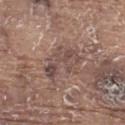Clinical impression: Recorded during total-body skin imaging; not selected for excision or biopsy. Clinical summary: The tile uses white-light illumination. About 5 mm across. The total-body-photography lesion software estimated a footprint of about 11 mm², a shape eccentricity near 0.85, and two-axis asymmetry of about 0.35. The analysis additionally found a border-irregularity index near 6/10 and a peripheral color-asymmetry measure near 2. Located on the upper back. A 15 mm crop from a total-body photograph taken for skin-cancer surveillance. The patient is a male in their 80s.